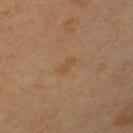The lesion was photographed on a routine skin check and not biopsied; there is no pathology result. Located on the left upper arm. Automated tile analysis of the lesion measured border irregularity of about 3.5 on a 0–10 scale and radial color variation of about 0. A 15 mm close-up tile from a total-body photography series done for melanoma screening. The patient is a female aged around 55. The recorded lesion diameter is about 2.5 mm.